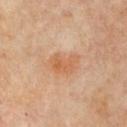Assessment: This lesion was catalogued during total-body skin photography and was not selected for biopsy. Clinical summary: Imaged with cross-polarized lighting. Cropped from a total-body skin-imaging series; the visible field is about 15 mm. Located on the front of the torso. A female patient aged approximately 65. The lesion-visualizer software estimated an area of roughly 6.5 mm² and a symmetry-axis asymmetry near 0.35. The analysis additionally found a color-variation rating of about 3/10 and a peripheral color-asymmetry measure near 1.